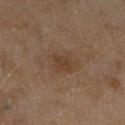Part of a total-body skin-imaging series; this lesion was reviewed on a skin check and was not flagged for biopsy.
A female subject approximately 60 years of age.
Cropped from a total-body skin-imaging series; the visible field is about 15 mm.
Automated tile analysis of the lesion measured an area of roughly 4.5 mm², an eccentricity of roughly 0.7, and a shape-asymmetry score of about 0.2 (0 = symmetric). The software also gave a border-irregularity rating of about 2/10, a color-variation rating of about 1/10, and a peripheral color-asymmetry measure near 0.5.
The lesion is on the left lower leg.
This is a cross-polarized tile.